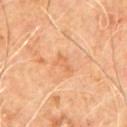- workup: no biopsy performed (imaged during a skin exam)
- automated metrics: a lesion color around L≈63 a*≈25 b*≈40 in CIELAB, a lesion–skin lightness drop of about 7, and a lesion-to-skin contrast of about 5 (normalized; higher = more distinct)
- patient: male, in their 60s
- image: ~15 mm tile from a whole-body skin photo
- lesion size: ~2.5 mm (longest diameter)
- site: the upper back
- lighting: cross-polarized illumination